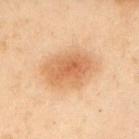Notes:
- anatomic site · the left upper arm
- lesion size · ~6.5 mm (longest diameter)
- imaging modality · total-body-photography crop, ~15 mm field of view
- subject · male, in their mid-50s